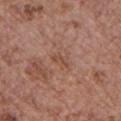biopsy status: total-body-photography surveillance lesion; no biopsy | patient: female, aged around 65 | illumination: white-light illumination | size: about 3 mm | site: the chest | imaging modality: 15 mm crop, total-body photography.